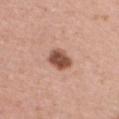Assessment:
No biopsy was performed on this lesion — it was imaged during a full skin examination and was not determined to be concerning.
Acquisition and patient details:
The recorded lesion diameter is about 3 mm. A close-up tile cropped from a whole-body skin photograph, about 15 mm across. From the chest. Captured under white-light illumination. A female subject, aged around 40.